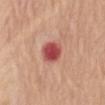image = ~15 mm tile from a whole-body skin photo | anatomic site = the abdomen | subject = female, aged 68–72.